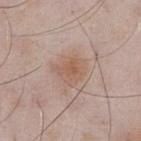Clinical impression:
Imaged during a routine full-body skin examination; the lesion was not biopsied and no histopathology is available.
Image and clinical context:
On the chest. A male subject aged around 55. A 15 mm close-up tile from a total-body photography series done for melanoma screening.